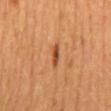Notes:
* workup: total-body-photography surveillance lesion; no biopsy
* patient: female
* body site: the back
* lighting: cross-polarized illumination
* diameter: ≈3 mm
* image: ~15 mm crop, total-body skin-cancer survey
* image-analysis metrics: a mean CIELAB color near L≈50 a*≈26 b*≈39, a lesion–skin lightness drop of about 12, and a normalized border contrast of about 8